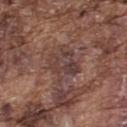biopsy status=no biopsy performed (imaged during a skin exam)
imaging modality=~15 mm crop, total-body skin-cancer survey
automated lesion analysis=a lesion area of about 10 mm², an eccentricity of roughly 0.6, and two-axis asymmetry of about 0.25; a color-variation rating of about 5/10 and a peripheral color-asymmetry measure near 1.5; an automated nevus-likeness rating near 0 out of 100 and lesion-presence confidence of about 70/100
subject=male, in their mid- to late 70s
location=the upper back